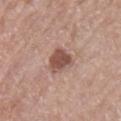biopsy status: total-body-photography surveillance lesion; no biopsy | lesion size: about 3.5 mm | patient: male, aged 68–72 | acquisition: ~15 mm tile from a whole-body skin photo | lighting: white-light illumination | site: the left upper arm.